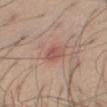Assessment: This lesion was catalogued during total-body skin photography and was not selected for biopsy. Background: The lesion is located on the right thigh. A male patient in their mid- to late 50s. A lesion tile, about 15 mm wide, cut from a 3D total-body photograph. The recorded lesion diameter is about 2.5 mm.